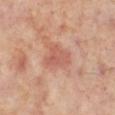Q: Is there a histopathology result?
A: total-body-photography surveillance lesion; no biopsy
Q: Who is the patient?
A: female, approximately 55 years of age
Q: What is the anatomic site?
A: the right lower leg
Q: Illumination type?
A: cross-polarized
Q: What kind of image is this?
A: total-body-photography crop, ~15 mm field of view